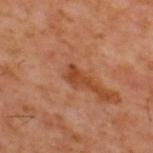This lesion was catalogued during total-body skin photography and was not selected for biopsy. A male subject in their 60s. The tile uses cross-polarized illumination. Longest diameter approximately 3 mm. Located on the upper back. A close-up tile cropped from a whole-body skin photograph, about 15 mm across.